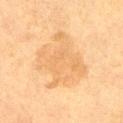Q: Is there a histopathology result?
A: catalogued during a skin exam; not biopsied
Q: What is the lesion's diameter?
A: ≈7.5 mm
Q: Lesion location?
A: the right thigh
Q: What is the imaging modality?
A: ~15 mm crop, total-body skin-cancer survey
Q: Who is the patient?
A: female, roughly 40 years of age